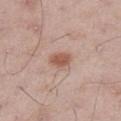biopsy status — catalogued during a skin exam; not biopsied
anatomic site — the right thigh
diameter — ≈2.5 mm
imaging modality — ~15 mm crop, total-body skin-cancer survey
tile lighting — white-light
automated metrics — a lesion color around L≈55 a*≈22 b*≈28 in CIELAB, about 11 CIELAB-L* units darker than the surrounding skin, and a normalized border contrast of about 8.5; lesion-presence confidence of about 100/100
patient — male, roughly 50 years of age